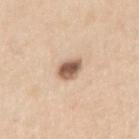No biopsy was performed on this lesion — it was imaged during a full skin examination and was not determined to be concerning. The tile uses white-light illumination. About 3 mm across. This image is a 15 mm lesion crop taken from a total-body photograph. From the right upper arm. A male subject roughly 60 years of age.